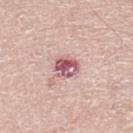Q: Was this lesion biopsied?
A: no biopsy performed (imaged during a skin exam)
Q: What is the anatomic site?
A: the left lower leg
Q: How was this image acquired?
A: total-body-photography crop, ~15 mm field of view
Q: Who is the patient?
A: male, aged 68 to 72